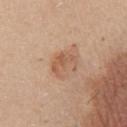biopsy_status: not biopsied; imaged during a skin examination
site: chest
image:
  source: total-body photography crop
  field_of_view_mm: 15
patient:
  sex: male
  age_approx: 40
lighting: white-light
lesion_size:
  long_diameter_mm_approx: 3.0